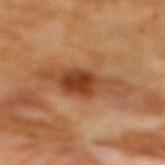Part of a total-body skin-imaging series; this lesion was reviewed on a skin check and was not flagged for biopsy. A 15 mm crop from a total-body photograph taken for skin-cancer surveillance. A female patient, about 55 years old. The lesion is on the mid back.